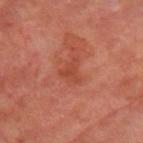– workup · catalogued during a skin exam; not biopsied
– patient · male, approximately 65 years of age
– anatomic site · the arm
– image source · ~15 mm crop, total-body skin-cancer survey
– TBP lesion metrics · a lesion color around L≈45 a*≈30 b*≈33 in CIELAB and roughly 6 lightness units darker than nearby skin; a border-irregularity rating of about 5.5/10, a within-lesion color-variation index near 2.5/10, and radial color variation of about 1; a lesion-detection confidence of about 100/100
– size · ≈3 mm